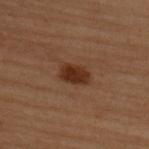Q: Is there a histopathology result?
A: imaged on a skin check; not biopsied
Q: What is the anatomic site?
A: the upper back
Q: How was this image acquired?
A: total-body-photography crop, ~15 mm field of view
Q: Patient demographics?
A: female, approximately 50 years of age